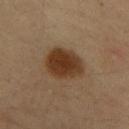workup: total-body-photography surveillance lesion; no biopsy | automated metrics: two-axis asymmetry of about 0.15; a border-irregularity index near 1.5/10, a color-variation rating of about 3/10, and radial color variation of about 1; a nevus-likeness score of about 100/100 and lesion-presence confidence of about 100/100 | subject: male, about 35 years old | diameter: ≈5.5 mm | anatomic site: the upper back | acquisition: 15 mm crop, total-body photography.